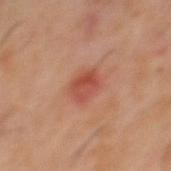workup: imaged on a skin check; not biopsied
acquisition: 15 mm crop, total-body photography
lighting: cross-polarized
subject: male, in their 60s
body site: the mid back
diameter: ≈3 mm
TBP lesion metrics: an average lesion color of about L≈45 a*≈28 b*≈30 (CIELAB), about 9 CIELAB-L* units darker than the surrounding skin, and a lesion-to-skin contrast of about 7 (normalized; higher = more distinct); a border-irregularity index near 2/10, internal color variation of about 4.5 on a 0–10 scale, and a peripheral color-asymmetry measure near 1.5; a nevus-likeness score of about 95/100 and a lesion-detection confidence of about 100/100